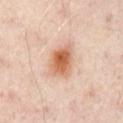{
  "biopsy_status": "not biopsied; imaged during a skin examination",
  "lesion_size": {
    "long_diameter_mm_approx": 4.0
  },
  "lighting": "cross-polarized",
  "automated_metrics": {
    "border_irregularity_0_10": 2.5,
    "nevus_likeness_0_100": 100,
    "lesion_detection_confidence_0_100": 100
  },
  "site": "abdomen",
  "patient": {
    "sex": "male",
    "age_approx": 50
  },
  "image": {
    "source": "total-body photography crop",
    "field_of_view_mm": 15
  }
}